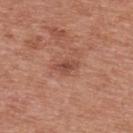Notes:
- biopsy status · no biopsy performed (imaged during a skin exam)